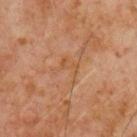Q: Is there a histopathology result?
A: no biopsy performed (imaged during a skin exam)
Q: How large is the lesion?
A: ~3 mm (longest diameter)
Q: How was this image acquired?
A: ~15 mm tile from a whole-body skin photo
Q: What did automated image analysis measure?
A: a mean CIELAB color near L≈50 a*≈21 b*≈35, about 5 CIELAB-L* units darker than the surrounding skin, and a lesion-to-skin contrast of about 4.5 (normalized; higher = more distinct); a border-irregularity rating of about 9/10, a within-lesion color-variation index near 1.5/10, and radial color variation of about 0.5; an automated nevus-likeness rating near 0 out of 100 and a lesion-detection confidence of about 100/100
Q: What are the patient's age and sex?
A: male, aged 58–62
Q: Illumination type?
A: cross-polarized
Q: What is the anatomic site?
A: the chest